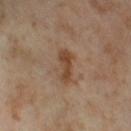Captured during whole-body skin photography for melanoma surveillance; the lesion was not biopsied. From the right thigh. The lesion's longest dimension is about 4.5 mm. A female subject approximately 55 years of age. Cropped from a whole-body photographic skin survey; the tile spans about 15 mm. The lesion-visualizer software estimated a border-irregularity rating of about 4/10, internal color variation of about 2 on a 0–10 scale, and radial color variation of about 0.5. This is a cross-polarized tile.